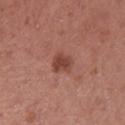Part of a total-body skin-imaging series; this lesion was reviewed on a skin check and was not flagged for biopsy.
Measured at roughly 2.5 mm in maximum diameter.
The patient is a female in their 40s.
The total-body-photography lesion software estimated an area of roughly 5 mm², an outline eccentricity of about 0.5 (0 = round, 1 = elongated), and a symmetry-axis asymmetry near 0.2. And it measured an average lesion color of about L≈44 a*≈26 b*≈27 (CIELAB), a lesion–skin lightness drop of about 10, and a normalized lesion–skin contrast near 7.5. The software also gave a nevus-likeness score of about 75/100.
On the right forearm.
The tile uses white-light illumination.
This image is a 15 mm lesion crop taken from a total-body photograph.